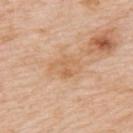The subject is a male aged approximately 75. This image is a 15 mm lesion crop taken from a total-body photograph. Captured under white-light illumination. About 3.5 mm across. From the back.